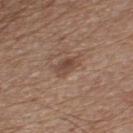  biopsy_status: not biopsied; imaged during a skin examination
  image:
    source: total-body photography crop
    field_of_view_mm: 15
  patient:
    sex: male
    age_approx: 75
  lighting: white-light
  site: chest
  lesion_size:
    long_diameter_mm_approx: 3.0
  automated_metrics:
    vs_skin_darker_L: 8.0
    vs_skin_contrast_norm: 6.5
    border_irregularity_0_10: 2.5
    color_variation_0_10: 3.5
    peripheral_color_asymmetry: 1.0
    nevus_likeness_0_100: 0
    lesion_detection_confidence_0_100: 100